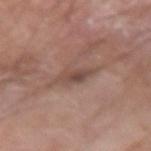From the right forearm. The tile uses white-light illumination. Automated image analysis of the tile measured a shape-asymmetry score of about 0.25 (0 = symmetric). It also reported a within-lesion color-variation index near 4/10. It also reported a classifier nevus-likeness of about 0/100 and lesion-presence confidence of about 90/100. A close-up tile cropped from a whole-body skin photograph, about 15 mm across. Measured at roughly 3.5 mm in maximum diameter. A male patient in their 80s.